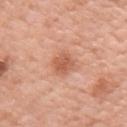Impression:
This lesion was catalogued during total-body skin photography and was not selected for biopsy.
Clinical summary:
From the right upper arm. The patient is a female aged 53 to 57. A roughly 15 mm field-of-view crop from a total-body skin photograph.